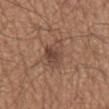Recorded during total-body skin imaging; not selected for excision or biopsy.
The recorded lesion diameter is about 4 mm.
On the mid back.
The tile uses white-light illumination.
A male patient aged around 65.
A 15 mm close-up tile from a total-body photography series done for melanoma screening.